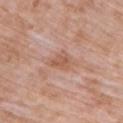workup: no biopsy performed (imaged during a skin exam) | automated lesion analysis: a footprint of about 5.5 mm², a shape eccentricity near 0.7, and a shape-asymmetry score of about 0.4 (0 = symmetric); an automated nevus-likeness rating near 0 out of 100 | patient: female, about 70 years old | site: the upper back | image: total-body-photography crop, ~15 mm field of view | lighting: white-light illumination | lesion diameter: about 3.5 mm.